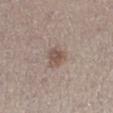Findings:
• workup · total-body-photography surveillance lesion; no biopsy
• acquisition · 15 mm crop, total-body photography
• patient · female, about 40 years old
• diameter · ≈3 mm
• illumination · white-light illumination
• location · the left lower leg